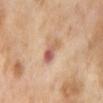follow-up — total-body-photography surveillance lesion; no biopsy
subject — female, aged approximately 55
location — the abdomen
lesion diameter — about 3.5 mm
image source — ~15 mm tile from a whole-body skin photo
tile lighting — cross-polarized
automated metrics — a mean CIELAB color near L≈61 a*≈23 b*≈30, roughly 12 lightness units darker than nearby skin, and a normalized lesion–skin contrast near 7.5; a color-variation rating of about 10/10 and a peripheral color-asymmetry measure near 3.5